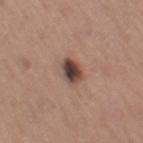Q: Was this lesion biopsied?
A: imaged on a skin check; not biopsied
Q: Lesion location?
A: the right thigh
Q: Automated lesion metrics?
A: an average lesion color of about L≈43 a*≈17 b*≈22 (CIELAB), roughly 16 lightness units darker than nearby skin, and a normalized border contrast of about 12.5
Q: Who is the patient?
A: female, aged approximately 65
Q: How was the tile lit?
A: white-light illumination
Q: What kind of image is this?
A: ~15 mm crop, total-body skin-cancer survey